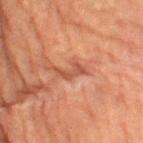Q: Was a biopsy performed?
A: total-body-photography surveillance lesion; no biopsy
Q: What is the lesion's diameter?
A: about 3 mm
Q: Who is the patient?
A: female, in their 80s
Q: How was this image acquired?
A: ~15 mm tile from a whole-body skin photo
Q: Lesion location?
A: the right lower leg
Q: How was the tile lit?
A: cross-polarized illumination